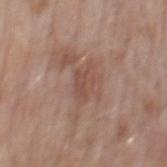Q: Is there a histopathology result?
A: imaged on a skin check; not biopsied
Q: What did automated image analysis measure?
A: a footprint of about 5 mm², an eccentricity of roughly 0.8, and two-axis asymmetry of about 0.3; a detector confidence of about 100 out of 100 that the crop contains a lesion
Q: Patient demographics?
A: male, aged 73–77
Q: How was this image acquired?
A: ~15 mm tile from a whole-body skin photo
Q: How large is the lesion?
A: ~3.5 mm (longest diameter)
Q: Illumination type?
A: white-light illumination
Q: Lesion location?
A: the mid back